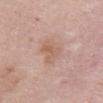Q: Was this lesion biopsied?
A: imaged on a skin check; not biopsied